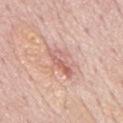Captured during whole-body skin photography for melanoma surveillance; the lesion was not biopsied.
A male patient, in their mid-70s.
From the mid back.
This is a white-light tile.
Cropped from a total-body skin-imaging series; the visible field is about 15 mm.